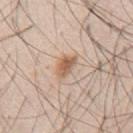Captured during whole-body skin photography for melanoma surveillance; the lesion was not biopsied. Automated tile analysis of the lesion measured a mean CIELAB color near L≈60 a*≈19 b*≈31, roughly 12 lightness units darker than nearby skin, and a lesion-to-skin contrast of about 8.5 (normalized; higher = more distinct). The analysis additionally found a border-irregularity index near 2.5/10, a within-lesion color-variation index near 3.5/10, and radial color variation of about 1. It also reported a nevus-likeness score of about 95/100 and lesion-presence confidence of about 100/100. From the mid back. A male subject in their mid- to late 40s. A roughly 15 mm field-of-view crop from a total-body skin photograph. This is a white-light tile. Approximately 3 mm at its widest.